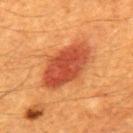Findings:
* biopsy status · imaged on a skin check; not biopsied
* patient · male, aged 58–62
* automated metrics · a lesion area of about 21 mm², an eccentricity of roughly 0.85, and a symmetry-axis asymmetry near 0.15; a mean CIELAB color near L≈48 a*≈33 b*≈39 and a lesion-to-skin contrast of about 9.5 (normalized; higher = more distinct); border irregularity of about 2 on a 0–10 scale and a within-lesion color-variation index near 5/10; an automated nevus-likeness rating near 100 out of 100 and a lesion-detection confidence of about 100/100
* acquisition · ~15 mm tile from a whole-body skin photo
* lighting · cross-polarized
* anatomic site · the back
* lesion diameter · ~7 mm (longest diameter)